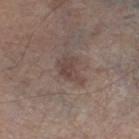Clinical impression:
The lesion was tiled from a total-body skin photograph and was not biopsied.
Clinical summary:
A lesion tile, about 15 mm wide, cut from a 3D total-body photograph. From the left thigh. The subject is a male approximately 75 years of age. The lesion-visualizer software estimated an outline eccentricity of about 0.75 (0 = round, 1 = elongated) and two-axis asymmetry of about 0.45. It also reported an average lesion color of about L≈43 a*≈15 b*≈21 (CIELAB), roughly 8 lightness units darker than nearby skin, and a normalized lesion–skin contrast near 6.5.